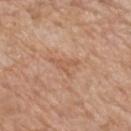Part of a total-body skin-imaging series; this lesion was reviewed on a skin check and was not flagged for biopsy. A 15 mm close-up tile from a total-body photography series done for melanoma screening. A male subject, in their 60s. Located on the mid back.